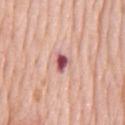Notes:
• workup: total-body-photography surveillance lesion; no biopsy
• subject: male, roughly 80 years of age
• TBP lesion metrics: a mean CIELAB color near L≈54 a*≈29 b*≈20; a border-irregularity rating of about 3.5/10, a within-lesion color-variation index near 4/10, and radial color variation of about 1.5; an automated nevus-likeness rating near 0 out of 100 and lesion-presence confidence of about 100/100
• image: total-body-photography crop, ~15 mm field of view
• anatomic site: the chest
• lighting: white-light